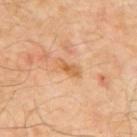• biopsy status: total-body-photography surveillance lesion; no biopsy
• acquisition: ~15 mm tile from a whole-body skin photo
• illumination: cross-polarized
• site: the mid back
• patient: male, aged approximately 55
• lesion size: ≈3.5 mm